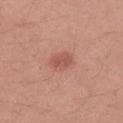Clinical impression:
The lesion was photographed on a routine skin check and not biopsied; there is no pathology result.
Acquisition and patient details:
From the right upper arm. A male subject, aged approximately 30. This image is a 15 mm lesion crop taken from a total-body photograph. Imaged with white-light lighting. Measured at roughly 3 mm in maximum diameter.